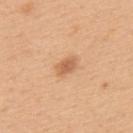<case>
<patient>
  <sex>male</sex>
  <age_approx>50</age_approx>
</patient>
<image>
  <source>total-body photography crop</source>
  <field_of_view_mm>15</field_of_view_mm>
</image>
<lighting>white-light</lighting>
<site>back</site>
<lesion_size>
  <long_diameter_mm_approx>3.0</long_diameter_mm_approx>
</lesion_size>
<automated_metrics>
  <cielab_L>62</cielab_L>
  <cielab_a>23</cielab_a>
  <cielab_b>38</cielab_b>
  <vs_skin_darker_L>11.0</vs_skin_darker_L>
  <vs_skin_contrast_norm>7.0</vs_skin_contrast_norm>
</automated_metrics>
</case>